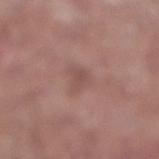The subject is a male aged around 65. Located on the right lower leg. Measured at roughly 3 mm in maximum diameter. This image is a 15 mm lesion crop taken from a total-body photograph. Imaged with white-light lighting.